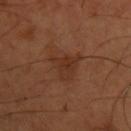Assessment: This lesion was catalogued during total-body skin photography and was not selected for biopsy. Clinical summary: Located on the upper back. Approximately 4 mm at its widest. An algorithmic analysis of the crop reported a normalized border contrast of about 6. The analysis additionally found a color-variation rating of about 3/10. Cropped from a whole-body photographic skin survey; the tile spans about 15 mm. A male subject, aged around 55. Captured under cross-polarized illumination.